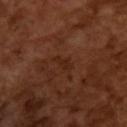Part of a total-body skin-imaging series; this lesion was reviewed on a skin check and was not flagged for biopsy. A male patient, approximately 65 years of age. Cropped from a total-body skin-imaging series; the visible field is about 15 mm.